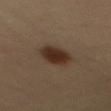{
  "biopsy_status": "not biopsied; imaged during a skin examination",
  "patient": {
    "sex": "female",
    "age_approx": 50
  },
  "lighting": "cross-polarized",
  "automated_metrics": {
    "area_mm2_approx": 9.0,
    "eccentricity": 0.8,
    "shape_asymmetry": 0.2,
    "nevus_likeness_0_100": 100
  },
  "image": {
    "source": "total-body photography crop",
    "field_of_view_mm": 15
  },
  "lesion_size": {
    "long_diameter_mm_approx": 4.5
  }
}